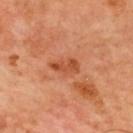<record>
<biopsy_status>not biopsied; imaged during a skin examination</biopsy_status>
<patient>
  <age_approx>65</age_approx>
</patient>
<lighting>cross-polarized</lighting>
<image>
  <source>total-body photography crop</source>
  <field_of_view_mm>15</field_of_view_mm>
</image>
<site>mid back</site>
<automated_metrics>
  <area_mm2_approx>4.5</area_mm2_approx>
  <eccentricity>0.9</eccentricity>
  <shape_asymmetry>0.35</shape_asymmetry>
  <cielab_L>50</cielab_L>
  <cielab_a>30</cielab_a>
  <cielab_b>39</cielab_b>
  <vs_skin_contrast_norm>7.5</vs_skin_contrast_norm>
  <nevus_likeness_0_100>0</nevus_likeness_0_100>
  <lesion_detection_confidence_0_100>100</lesion_detection_confidence_0_100>
</automated_metrics>
</record>